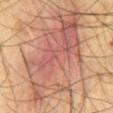- follow-up · total-body-photography surveillance lesion; no biopsy
- body site · the front of the torso
- image-analysis metrics · a footprint of about 55 mm², an eccentricity of roughly 0.85, and a symmetry-axis asymmetry near 0.35; border irregularity of about 6.5 on a 0–10 scale, a color-variation rating of about 7/10, and peripheral color asymmetry of about 2; an automated nevus-likeness rating near 35 out of 100
- lesion diameter · about 13 mm
- tile lighting · cross-polarized
- imaging modality · total-body-photography crop, ~15 mm field of view
- subject · male, about 65 years old